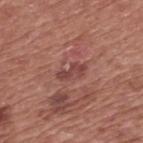Captured during whole-body skin photography for melanoma surveillance; the lesion was not biopsied. A close-up tile cropped from a whole-body skin photograph, about 15 mm across. Located on the back. A male patient aged approximately 75.